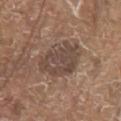This lesion was catalogued during total-body skin photography and was not selected for biopsy. The recorded lesion diameter is about 6 mm. Cropped from a total-body skin-imaging series; the visible field is about 15 mm. The lesion is located on the arm. The patient is a male aged approximately 80.